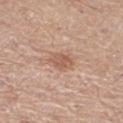This lesion was catalogued during total-body skin photography and was not selected for biopsy. The lesion's longest dimension is about 2.5 mm. The lesion-visualizer software estimated a border-irregularity index near 3/10, internal color variation of about 2.5 on a 0–10 scale, and a peripheral color-asymmetry measure near 1. The analysis additionally found a lesion-detection confidence of about 100/100. On the right thigh. Imaged with white-light lighting. This image is a 15 mm lesion crop taken from a total-body photograph. A female subject, in their 50s.